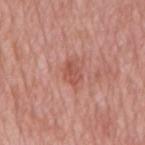Recorded during total-body skin imaging; not selected for excision or biopsy. A 15 mm crop from a total-body photograph taken for skin-cancer surveillance. The lesion is on the mid back. A male subject aged approximately 65.